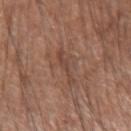Captured during whole-body skin photography for melanoma surveillance; the lesion was not biopsied. Longest diameter approximately 3.5 mm. A lesion tile, about 15 mm wide, cut from a 3D total-body photograph. A male subject, aged 53–57. The lesion is located on the right forearm.